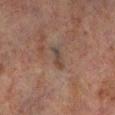lighting: cross-polarized
patient:
  sex: male
  age_approx: 70
image:
  source: total-body photography crop
  field_of_view_mm: 15
lesion_size:
  long_diameter_mm_approx: 3.0
site: left lower leg